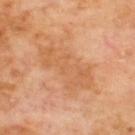Part of a total-body skin-imaging series; this lesion was reviewed on a skin check and was not flagged for biopsy.
On the upper back.
A close-up tile cropped from a whole-body skin photograph, about 15 mm across.
A male subject aged 68–72.
Measured at roughly 8 mm in maximum diameter.